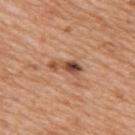Part of a total-body skin-imaging series; this lesion was reviewed on a skin check and was not flagged for biopsy. The lesion-visualizer software estimated a lesion area of about 5 mm², an outline eccentricity of about 0.95 (0 = round, 1 = elongated), and a symmetry-axis asymmetry near 0.4. The software also gave a lesion color around L≈50 a*≈23 b*≈32 in CIELAB and about 14 CIELAB-L* units darker than the surrounding skin. It also reported border irregularity of about 5 on a 0–10 scale and a within-lesion color-variation index near 6/10. The software also gave a classifier nevus-likeness of about 75/100 and a detector confidence of about 100 out of 100 that the crop contains a lesion. Located on the right upper arm. Imaged with white-light lighting. The patient is a female aged approximately 55. A 15 mm close-up extracted from a 3D total-body photography capture. About 4 mm across.